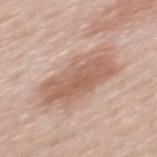Impression: Captured during whole-body skin photography for melanoma surveillance; the lesion was not biopsied. Image and clinical context: Cropped from a total-body skin-imaging series; the visible field is about 15 mm. Automated tile analysis of the lesion measured an area of roughly 24 mm² and an outline eccentricity of about 0.85 (0 = round, 1 = elongated). The software also gave a lesion color around L≈60 a*≈20 b*≈28 in CIELAB, about 11 CIELAB-L* units darker than the surrounding skin, and a normalized border contrast of about 7. The analysis additionally found border irregularity of about 3.5 on a 0–10 scale, a color-variation rating of about 4/10, and radial color variation of about 1.5. The lesion's longest dimension is about 7.5 mm. On the back. Captured under white-light illumination. A female subject, aged approximately 60.